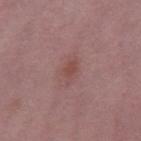tile lighting = white-light illumination
body site = the right thigh
size = about 3 mm
subject = female, about 40 years old
image = ~15 mm tile from a whole-body skin photo